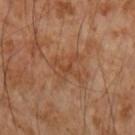workup = no biopsy performed (imaged during a skin exam); anatomic site = the right forearm; image source = ~15 mm crop, total-body skin-cancer survey; subject = male, roughly 55 years of age.